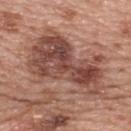Clinical impression:
Captured during whole-body skin photography for melanoma surveillance; the lesion was not biopsied.
Image and clinical context:
Located on the upper back. The lesion's longest dimension is about 10.5 mm. Cropped from a whole-body photographic skin survey; the tile spans about 15 mm. This is a white-light tile. The subject is a female aged 58–62. The lesion-visualizer software estimated a border-irregularity rating of about 6/10 and a color-variation rating of about 7.5/10.